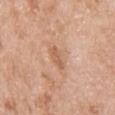  biopsy_status: not biopsied; imaged during a skin examination
  lesion_size:
    long_diameter_mm_approx: 3.0
  site: chest
  image:
    source: total-body photography crop
    field_of_view_mm: 15
  patient:
    sex: male
    age_approx: 80
  automated_metrics:
    cielab_L: 60
    cielab_a: 22
    cielab_b: 34
    vs_skin_darker_L: 8.0
  lighting: white-light